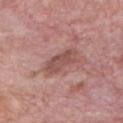Q: Is there a histopathology result?
A: catalogued during a skin exam; not biopsied
Q: Who is the patient?
A: male, aged around 60
Q: What is the anatomic site?
A: the chest
Q: How large is the lesion?
A: ≈4.5 mm
Q: How was this image acquired?
A: ~15 mm crop, total-body skin-cancer survey
Q: What lighting was used for the tile?
A: white-light illumination